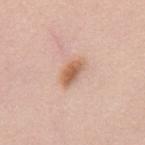biopsy status: total-body-photography surveillance lesion; no biopsy | diameter: ≈4 mm | tile lighting: white-light | patient: female, aged approximately 65 | location: the back | acquisition: total-body-photography crop, ~15 mm field of view.